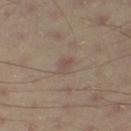Q: Was this lesion biopsied?
A: catalogued during a skin exam; not biopsied
Q: Patient demographics?
A: male, aged around 55
Q: How large is the lesion?
A: about 2.5 mm
Q: What kind of image is this?
A: total-body-photography crop, ~15 mm field of view
Q: How was the tile lit?
A: cross-polarized
Q: Automated lesion metrics?
A: a shape eccentricity near 0.85 and a shape-asymmetry score of about 0.45 (0 = symmetric); border irregularity of about 4 on a 0–10 scale, a color-variation rating of about 1/10, and peripheral color asymmetry of about 0.5; a nevus-likeness score of about 0/100 and lesion-presence confidence of about 100/100
Q: Where on the body is the lesion?
A: the right thigh